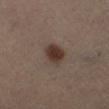Part of a total-body skin-imaging series; this lesion was reviewed on a skin check and was not flagged for biopsy. The lesion is on the left leg. A 15 mm crop from a total-body photograph taken for skin-cancer surveillance. The recorded lesion diameter is about 3 mm. The lesion-visualizer software estimated a mean CIELAB color near L≈33 a*≈14 b*≈21, roughly 11 lightness units darker than nearby skin, and a lesion-to-skin contrast of about 10.5 (normalized; higher = more distinct). The tile uses cross-polarized illumination. A male patient about 50 years old.